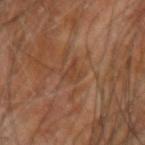The lesion was photographed on a routine skin check and not biopsied; there is no pathology result. On the right forearm. The lesion's longest dimension is about 3 mm. A male subject, aged 63 to 67. A 15 mm close-up extracted from a 3D total-body photography capture.